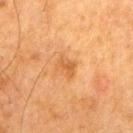<tbp_lesion>
<biopsy_status>not biopsied; imaged during a skin examination</biopsy_status>
<lighting>cross-polarized</lighting>
<automated_metrics>
  <eccentricity>0.75</eccentricity>
  <vs_skin_darker_L>7.0</vs_skin_darker_L>
  <border_irregularity_0_10>3.0</border_irregularity_0_10>
  <color_variation_0_10>2.5</color_variation_0_10>
  <peripheral_color_asymmetry>1.0</peripheral_color_asymmetry>
  <lesion_detection_confidence_0_100>100</lesion_detection_confidence_0_100>
</automated_metrics>
<patient>
  <sex>male</sex>
  <age_approx>80</age_approx>
</patient>
<image>
  <source>total-body photography crop</source>
  <field_of_view_mm>15</field_of_view_mm>
</image>
<site>leg</site>
<lesion_size>
  <long_diameter_mm_approx>2.5</long_diameter_mm_approx>
</lesion_size>
</tbp_lesion>